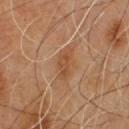Impression:
This lesion was catalogued during total-body skin photography and was not selected for biopsy.
Acquisition and patient details:
On the chest. Imaged with cross-polarized lighting. A male patient in their mid-50s. The lesion's longest dimension is about 4 mm. This image is a 15 mm lesion crop taken from a total-body photograph.